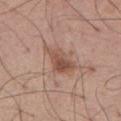Imaged with white-light lighting. A roughly 15 mm field-of-view crop from a total-body skin photograph. Approximately 4.5 mm at its widest. Automated image analysis of the tile measured a lesion area of about 9 mm² and an outline eccentricity of about 0.8 (0 = round, 1 = elongated). And it measured a lesion color around L≈52 a*≈20 b*≈27 in CIELAB, about 10 CIELAB-L* units darker than the surrounding skin, and a normalized lesion–skin contrast near 7. The subject is a male aged 63 to 67. Located on the abdomen.